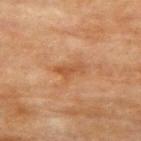Automated tile analysis of the lesion measured a shape eccentricity near 0.85 and a symmetry-axis asymmetry near 0.4. The software also gave an average lesion color of about L≈46 a*≈21 b*≈34 (CIELAB) and about 7 CIELAB-L* units darker than the surrounding skin. It also reported a border-irregularity index near 4.5/10, internal color variation of about 1.5 on a 0–10 scale, and a peripheral color-asymmetry measure near 0.5. The analysis additionally found an automated nevus-likeness rating near 0 out of 100 and a lesion-detection confidence of about 100/100. Imaged with cross-polarized lighting. The subject is a female aged around 80. Cropped from a total-body skin-imaging series; the visible field is about 15 mm. Located on the upper back. Longest diameter approximately 3.5 mm.